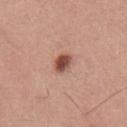Assessment:
Part of a total-body skin-imaging series; this lesion was reviewed on a skin check and was not flagged for biopsy.
Image and clinical context:
The tile uses white-light illumination. A region of skin cropped from a whole-body photographic capture, roughly 15 mm wide. Automated image analysis of the tile measured a shape-asymmetry score of about 0.15 (0 = symmetric). It also reported an average lesion color of about L≈50 a*≈23 b*≈27 (CIELAB) and a normalized lesion–skin contrast near 10.5. The analysis additionally found internal color variation of about 3.5 on a 0–10 scale and a peripheral color-asymmetry measure near 1. The lesion is located on the chest. About 3 mm across. The patient is a male aged 38–42.